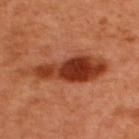Clinical summary: A male subject aged 48–52. An algorithmic analysis of the crop reported a lesion area of about 20 mm² and a shape-asymmetry score of about 0.35 (0 = symmetric). The software also gave about 15 CIELAB-L* units darker than the surrounding skin and a lesion-to-skin contrast of about 11 (normalized; higher = more distinct). It also reported border irregularity of about 5.5 on a 0–10 scale, internal color variation of about 7 on a 0–10 scale, and peripheral color asymmetry of about 2.5. It also reported a classifier nevus-likeness of about 95/100 and a lesion-detection confidence of about 100/100. Cropped from a total-body skin-imaging series; the visible field is about 15 mm. The lesion is located on the back. The tile uses cross-polarized illumination.